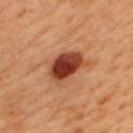Q: Was a biopsy performed?
A: no biopsy performed (imaged during a skin exam)
Q: How was this image acquired?
A: 15 mm crop, total-body photography
Q: Where on the body is the lesion?
A: the upper back
Q: What lighting was used for the tile?
A: cross-polarized
Q: What are the patient's age and sex?
A: male, in their 50s
Q: What did automated image analysis measure?
A: an area of roughly 12 mm² and an eccentricity of roughly 0.75; a classifier nevus-likeness of about 100/100 and a detector confidence of about 100 out of 100 that the crop contains a lesion